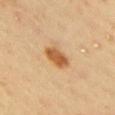Findings:
* biopsy status · no biopsy performed (imaged during a skin exam)
* patient · male, roughly 40 years of age
* anatomic site · the right upper arm
* image source · ~15 mm tile from a whole-body skin photo
* illumination · cross-polarized
* lesion diameter · ≈3 mm
* automated metrics · an average lesion color of about L≈57 a*≈24 b*≈41 (CIELAB), roughly 14 lightness units darker than nearby skin, and a lesion-to-skin contrast of about 9.5 (normalized; higher = more distinct); a border-irregularity rating of about 2/10, internal color variation of about 3 on a 0–10 scale, and a peripheral color-asymmetry measure near 1; a nevus-likeness score of about 100/100 and lesion-presence confidence of about 100/100